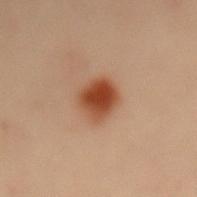A female patient, aged 48–52.
The lesion is on the mid back.
This is a cross-polarized tile.
The recorded lesion diameter is about 4 mm.
A region of skin cropped from a whole-body photographic capture, roughly 15 mm wide.